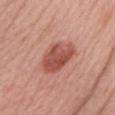Assessment:
Imaged during a routine full-body skin examination; the lesion was not biopsied and no histopathology is available.
Background:
A 15 mm close-up extracted from a 3D total-body photography capture. The lesion is on the chest. Imaged with white-light lighting. The patient is a female in their 60s. Approximately 5 mm at its widest. Automated tile analysis of the lesion measured a border-irregularity index near 2/10, internal color variation of about 4.5 on a 0–10 scale, and a peripheral color-asymmetry measure near 1.5. The analysis additionally found lesion-presence confidence of about 100/100.